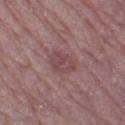No biopsy was performed on this lesion — it was imaged during a full skin examination and was not determined to be concerning. The lesion is located on the left thigh. A male subject, in their mid-70s. Cropped from a total-body skin-imaging series; the visible field is about 15 mm.